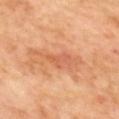Clinical impression: Captured during whole-body skin photography for melanoma surveillance; the lesion was not biopsied. Clinical summary: The tile uses cross-polarized illumination. From the upper back. A 15 mm close-up extracted from a 3D total-body photography capture. Measured at roughly 3.5 mm in maximum diameter.